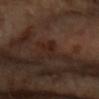biopsy status: imaged on a skin check; not biopsied | imaging modality: ~15 mm crop, total-body skin-cancer survey | patient: female, aged around 60 | lesion size: ≈3 mm | lighting: cross-polarized | location: the right forearm | automated metrics: a border-irregularity rating of about 6/10, internal color variation of about 0 on a 0–10 scale, and radial color variation of about 0; lesion-presence confidence of about 85/100.